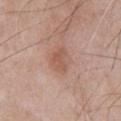Part of a total-body skin-imaging series; this lesion was reviewed on a skin check and was not flagged for biopsy.
Located on the chest.
A male patient approximately 50 years of age.
This is a white-light tile.
Longest diameter approximately 3.5 mm.
A 15 mm close-up tile from a total-body photography series done for melanoma screening.